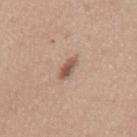Q: Is there a histopathology result?
A: imaged on a skin check; not biopsied
Q: What is the lesion's diameter?
A: ≈3 mm
Q: Illumination type?
A: white-light illumination
Q: Automated lesion metrics?
A: a classifier nevus-likeness of about 75/100 and a detector confidence of about 100 out of 100 that the crop contains a lesion
Q: What kind of image is this?
A: ~15 mm tile from a whole-body skin photo
Q: Where on the body is the lesion?
A: the mid back
Q: Patient demographics?
A: male, aged approximately 65